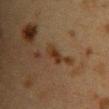The lesion was tiled from a total-body skin photograph and was not biopsied. The lesion is located on the chest. A female patient about 40 years old. This image is a 15 mm lesion crop taken from a total-body photograph.